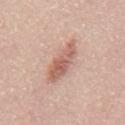This lesion was catalogued during total-body skin photography and was not selected for biopsy. The lesion-visualizer software estimated a lesion color around L≈61 a*≈22 b*≈27 in CIELAB and a lesion-to-skin contrast of about 7.5 (normalized; higher = more distinct). On the mid back. This image is a 15 mm lesion crop taken from a total-body photograph. A male subject, aged around 50. The tile uses white-light illumination.